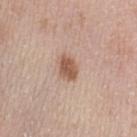Background:
Longest diameter approximately 3 mm. Automated tile analysis of the lesion measured a footprint of about 5 mm² and a shape-asymmetry score of about 0.25 (0 = symmetric). It also reported an average lesion color of about L≈55 a*≈20 b*≈30 (CIELAB), about 13 CIELAB-L* units darker than the surrounding skin, and a normalized lesion–skin contrast near 9. The analysis additionally found a border-irregularity index near 2/10, internal color variation of about 2.5 on a 0–10 scale, and peripheral color asymmetry of about 1. This is a white-light tile. The lesion is located on the left upper arm. A 15 mm close-up extracted from a 3D total-body photography capture. The patient is a female aged around 50.